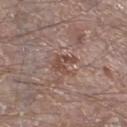Q: Is there a histopathology result?
A: no biopsy performed (imaged during a skin exam)
Q: Patient demographics?
A: male, aged around 65
Q: What did automated image analysis measure?
A: a border-irregularity rating of about 5/10, a within-lesion color-variation index near 2.5/10, and a peripheral color-asymmetry measure near 1.5
Q: Lesion size?
A: about 3 mm
Q: What kind of image is this?
A: total-body-photography crop, ~15 mm field of view
Q: How was the tile lit?
A: white-light illumination
Q: Where on the body is the lesion?
A: the left lower leg